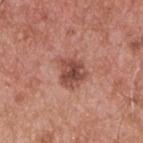workup — total-body-photography surveillance lesion; no biopsy
imaging modality — ~15 mm tile from a whole-body skin photo
subject — male, in their mid-50s
site — the upper back
image-analysis metrics — a shape eccentricity near 0.5 and a symmetry-axis asymmetry near 0.3; border irregularity of about 3 on a 0–10 scale, internal color variation of about 4.5 on a 0–10 scale, and radial color variation of about 1.5; a detector confidence of about 100 out of 100 that the crop contains a lesion
tile lighting — white-light
size — about 3.5 mm